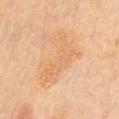<tbp_lesion>
<patient>
  <sex>female</sex>
  <age_approx>65</age_approx>
</patient>
<site>front of the torso</site>
<lighting>cross-polarized</lighting>
<image>
  <source>total-body photography crop</source>
  <field_of_view_mm>15</field_of_view_mm>
</image>
<lesion_size>
  <long_diameter_mm_approx>7.5</long_diameter_mm_approx>
</lesion_size>
</tbp_lesion>